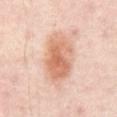Case summary:
• biopsy status: imaged on a skin check; not biopsied
• acquisition: ~15 mm crop, total-body skin-cancer survey
• subject: roughly 55 years of age
• anatomic site: the abdomen